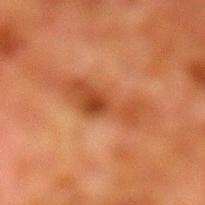Recorded during total-body skin imaging; not selected for excision or biopsy.
About 6 mm across.
A male patient roughly 80 years of age.
Automated image analysis of the tile measured an area of roughly 11 mm², an eccentricity of roughly 0.95, and two-axis asymmetry of about 0.4. The analysis additionally found a color-variation rating of about 3.5/10. The software also gave a classifier nevus-likeness of about 0/100 and a detector confidence of about 100 out of 100 that the crop contains a lesion.
Located on the left lower leg.
Cropped from a total-body skin-imaging series; the visible field is about 15 mm.
This is a cross-polarized tile.